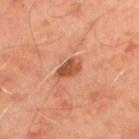The tile uses cross-polarized illumination.
The subject is a male roughly 50 years of age.
Approximately 2.5 mm at its widest.
Cropped from a whole-body photographic skin survey; the tile spans about 15 mm.
Located on the upper back.